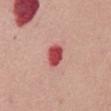Clinical impression:
This lesion was catalogued during total-body skin photography and was not selected for biopsy.
Clinical summary:
Imaged with white-light lighting. A female patient, roughly 65 years of age. The lesion is located on the abdomen. A 15 mm close-up extracted from a 3D total-body photography capture. Measured at roughly 2.5 mm in maximum diameter.